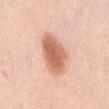Assessment: No biopsy was performed on this lesion — it was imaged during a full skin examination and was not determined to be concerning. Background: This image is a 15 mm lesion crop taken from a total-body photograph. The lesion is located on the abdomen. The tile uses white-light illumination. The lesion-visualizer software estimated an average lesion color of about L≈65 a*≈26 b*≈32 (CIELAB) and roughly 15 lightness units darker than nearby skin. The analysis additionally found a border-irregularity rating of about 2/10, a within-lesion color-variation index near 4.5/10, and peripheral color asymmetry of about 1. A female subject aged 38 to 42.